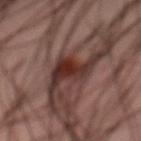Notes:
* workup — total-body-photography surveillance lesion; no biopsy
* site — the abdomen
* acquisition — ~15 mm crop, total-body skin-cancer survey
* lesion diameter — ≈7 mm
* image-analysis metrics — a footprint of about 10 mm², an outline eccentricity of about 0.85 (0 = round, 1 = elongated), and two-axis asymmetry of about 0.55; a detector confidence of about 95 out of 100 that the crop contains a lesion
* subject — male, roughly 45 years of age
* illumination — cross-polarized